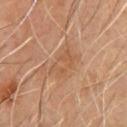Captured during whole-body skin photography for melanoma surveillance; the lesion was not biopsied. Longest diameter approximately 3.5 mm. This image is a 15 mm lesion crop taken from a total-body photograph. Automated image analysis of the tile measured an eccentricity of roughly 0.8. The software also gave border irregularity of about 3.5 on a 0–10 scale, internal color variation of about 3 on a 0–10 scale, and a peripheral color-asymmetry measure near 1. It also reported an automated nevus-likeness rating near 0 out of 100. The lesion is located on the chest. A male patient, aged approximately 55. This is a cross-polarized tile.